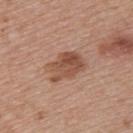A female subject roughly 45 years of age. On the left upper arm. The tile uses white-light illumination. Cropped from a total-body skin-imaging series; the visible field is about 15 mm.